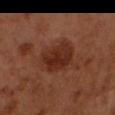| key | value |
|---|---|
| subject | male, aged approximately 50 |
| anatomic site | the head or neck |
| diameter | about 5 mm |
| tile lighting | cross-polarized |
| image source | total-body-photography crop, ~15 mm field of view |